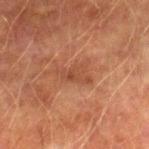Q: Was this lesion biopsied?
A: total-body-photography surveillance lesion; no biopsy
Q: What kind of image is this?
A: 15 mm crop, total-body photography
Q: What did automated image analysis measure?
A: a lesion color around L≈39 a*≈22 b*≈29 in CIELAB, roughly 6 lightness units darker than nearby skin, and a normalized lesion–skin contrast near 5.5; a classifier nevus-likeness of about 0/100 and a detector confidence of about 100 out of 100 that the crop contains a lesion
Q: How was the tile lit?
A: cross-polarized illumination
Q: Patient demographics?
A: male, about 75 years old
Q: Where on the body is the lesion?
A: the right lower leg